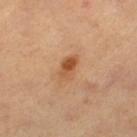biopsy_status: not biopsied; imaged during a skin examination
patient:
  sex: female
  age_approx: 50
site: left thigh
image:
  source: total-body photography crop
  field_of_view_mm: 15
lighting: cross-polarized